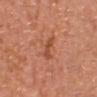{"biopsy_status": "not biopsied; imaged during a skin examination", "lesion_size": {"long_diameter_mm_approx": 2.5}, "patient": {"sex": "male", "age_approx": 40}, "automated_metrics": {"eccentricity": 0.85, "shape_asymmetry": 0.4, "cielab_L": 50, "cielab_a": 28, "cielab_b": 36, "vs_skin_darker_L": 8.0, "vs_skin_contrast_norm": 6.5, "nevus_likeness_0_100": 15, "lesion_detection_confidence_0_100": 100}, "site": "head or neck", "image": {"source": "total-body photography crop", "field_of_view_mm": 15}}